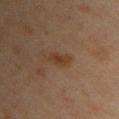The lesion was tiled from a total-body skin photograph and was not biopsied. Approximately 3 mm at its widest. A female subject, aged around 45. From the front of the torso. The lesion-visualizer software estimated a footprint of about 4 mm², an outline eccentricity of about 0.9 (0 = round, 1 = elongated), and a symmetry-axis asymmetry near 0.3. And it measured an average lesion color of about L≈32 a*≈15 b*≈27 (CIELAB), roughly 6 lightness units darker than nearby skin, and a lesion-to-skin contrast of about 7.5 (normalized; higher = more distinct). The analysis additionally found an automated nevus-likeness rating near 20 out of 100 and lesion-presence confidence of about 100/100. A lesion tile, about 15 mm wide, cut from a 3D total-body photograph. This is a cross-polarized tile.